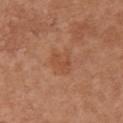biopsy_status: not biopsied; imaged during a skin examination
patient:
  sex: female
  age_approx: 65
site: chest
image:
  source: total-body photography crop
  field_of_view_mm: 15
lighting: white-light
lesion_size:
  long_diameter_mm_approx: 3.5